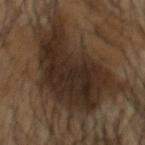Findings:
• notes · imaged on a skin check; not biopsied
• anatomic site · the head or neck
• subject · male, in their mid-50s
• image · 15 mm crop, total-body photography